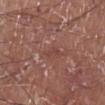<record>
<biopsy_status>not biopsied; imaged during a skin examination</biopsy_status>
<site>left lower leg</site>
<lesion_size>
  <long_diameter_mm_approx>2.5</long_diameter_mm_approx>
</lesion_size>
<image>
  <source>total-body photography crop</source>
  <field_of_view_mm>15</field_of_view_mm>
</image>
<lighting>white-light</lighting>
<patient>
  <sex>male</sex>
  <age_approx>55</age_approx>
</patient>
</record>